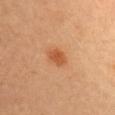Imaged during a routine full-body skin examination; the lesion was not biopsied and no histopathology is available. A female patient, aged 33–37. On the head or neck. Cropped from a total-body skin-imaging series; the visible field is about 15 mm. The tile uses cross-polarized illumination. Automated image analysis of the tile measured a footprint of about 4 mm², an eccentricity of roughly 0.75, and a shape-asymmetry score of about 0.25 (0 = symmetric). The analysis additionally found roughly 10 lightness units darker than nearby skin. And it measured a border-irregularity index near 2/10 and internal color variation of about 1.5 on a 0–10 scale. The analysis additionally found a nevus-likeness score of about 90/100 and a lesion-detection confidence of about 100/100. Approximately 2.5 mm at its widest.